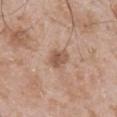* follow-up: no biopsy performed (imaged during a skin exam)
* acquisition: total-body-photography crop, ~15 mm field of view
* size: ~3 mm (longest diameter)
* automated metrics: a footprint of about 5 mm², a shape eccentricity near 0.75, and two-axis asymmetry of about 0.3; a normalized lesion–skin contrast near 7; border irregularity of about 2.5 on a 0–10 scale and a peripheral color-asymmetry measure near 1; a nevus-likeness score of about 20/100
* subject: male, in their 50s
* location: the mid back